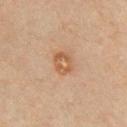Recorded during total-body skin imaging; not selected for excision or biopsy.